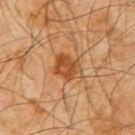- biopsy status — catalogued during a skin exam; not biopsied
- lighting — cross-polarized illumination
- patient — male, aged 58–62
- site — the arm
- imaging modality — 15 mm crop, total-body photography
- automated metrics — an area of roughly 8 mm², an outline eccentricity of about 0.6 (0 = round, 1 = elongated), and a shape-asymmetry score of about 0.25 (0 = symmetric)